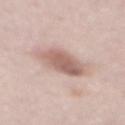<lesion>
<biopsy_status>not biopsied; imaged during a skin examination</biopsy_status>
<image>
  <source>total-body photography crop</source>
  <field_of_view_mm>15</field_of_view_mm>
</image>
<automated_metrics>
  <eccentricity>0.9</eccentricity>
  <shape_asymmetry>0.2</shape_asymmetry>
  <cielab_L>61</cielab_L>
  <cielab_a>19</cielab_a>
  <cielab_b>24</cielab_b>
  <vs_skin_darker_L>13.0</vs_skin_darker_L>
  <vs_skin_contrast_norm>8.0</vs_skin_contrast_norm>
</automated_metrics>
<patient>
  <sex>female</sex>
  <age_approx>50</age_approx>
</patient>
<lesion_size>
  <long_diameter_mm_approx>6.0</long_diameter_mm_approx>
</lesion_size>
<site>back</site>
<lighting>white-light</lighting>
</lesion>